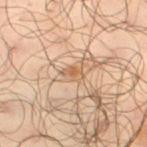<case>
<site>leg</site>
<patient>
  <sex>male</sex>
  <age_approx>65</age_approx>
</patient>
<image>
  <source>total-body photography crop</source>
  <field_of_view_mm>15</field_of_view_mm>
</image>
</case>